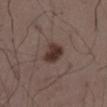Impression: Imaged during a routine full-body skin examination; the lesion was not biopsied and no histopathology is available. Clinical summary: Automated tile analysis of the lesion measured a lesion area of about 6.5 mm², an outline eccentricity of about 0.45 (0 = round, 1 = elongated), and a symmetry-axis asymmetry near 0.25. And it measured an average lesion color of about L≈34 a*≈15 b*≈20 (CIELAB) and a lesion-to-skin contrast of about 10.5 (normalized; higher = more distinct). The analysis additionally found a border-irregularity index near 2/10 and a color-variation rating of about 3.5/10. A male patient in their 50s. The recorded lesion diameter is about 3 mm. Captured under white-light illumination. A close-up tile cropped from a whole-body skin photograph, about 15 mm across. The lesion is located on the abdomen.